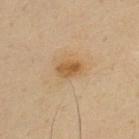The lesion was photographed on a routine skin check and not biopsied; there is no pathology result. Longest diameter approximately 3 mm. A close-up tile cropped from a whole-body skin photograph, about 15 mm across. The total-body-photography lesion software estimated a lesion color around L≈49 a*≈16 b*≈35 in CIELAB, about 9 CIELAB-L* units darker than the surrounding skin, and a lesion-to-skin contrast of about 8 (normalized; higher = more distinct). And it measured border irregularity of about 1.5 on a 0–10 scale. It also reported a nevus-likeness score of about 85/100 and a detector confidence of about 100 out of 100 that the crop contains a lesion. A male patient roughly 35 years of age. Imaged with cross-polarized lighting. The lesion is on the upper back.